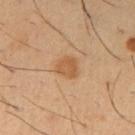The lesion was tiled from a total-body skin photograph and was not biopsied. Automated tile analysis of the lesion measured an outline eccentricity of about 0.4 (0 = round, 1 = elongated) and a shape-asymmetry score of about 0.3 (0 = symmetric). The software also gave a border-irregularity index near 2.5/10, internal color variation of about 2 on a 0–10 scale, and radial color variation of about 0.5. And it measured a classifier nevus-likeness of about 70/100 and a lesion-detection confidence of about 100/100. From the right upper arm. The patient is a male roughly 40 years of age. A region of skin cropped from a whole-body photographic capture, roughly 15 mm wide.